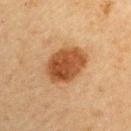This lesion was catalogued during total-body skin photography and was not selected for biopsy. On the right upper arm. The subject is a male approximately 65 years of age. Cropped from a whole-body photographic skin survey; the tile spans about 15 mm.